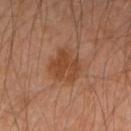<tbp_lesion>
  <lighting>cross-polarized</lighting>
  <patient>
    <sex>male</sex>
    <age_approx>45</age_approx>
  </patient>
  <lesion_size>
    <long_diameter_mm_approx>4.0</long_diameter_mm_approx>
  </lesion_size>
  <site>left forearm</site>
  <image>
    <source>total-body photography crop</source>
    <field_of_view_mm>15</field_of_view_mm>
  </image>
</tbp_lesion>